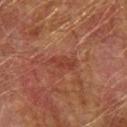Impression:
The lesion was photographed on a routine skin check and not biopsied; there is no pathology result.
Context:
This is a cross-polarized tile. The lesion is on the left forearm. Measured at roughly 3.5 mm in maximum diameter. Cropped from a whole-body photographic skin survey; the tile spans about 15 mm. A male subject approximately 75 years of age.